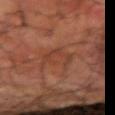biopsy status — imaged on a skin check; not biopsied
image — ~15 mm tile from a whole-body skin photo
subject — male, about 60 years old
anatomic site — the arm